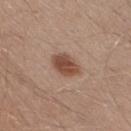notes: imaged on a skin check; not biopsied | body site: the right thigh | patient: male, in their 30s | automated metrics: an eccentricity of roughly 0.75 and two-axis asymmetry of about 0.15 | lighting: white-light illumination | acquisition: ~15 mm tile from a whole-body skin photo.